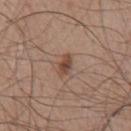<record>
<biopsy_status>not biopsied; imaged during a skin examination</biopsy_status>
<site>chest</site>
<image>
  <source>total-body photography crop</source>
  <field_of_view_mm>15</field_of_view_mm>
</image>
<lesion_size>
  <long_diameter_mm_approx>2.5</long_diameter_mm_approx>
</lesion_size>
<patient>
  <sex>male</sex>
  <age_approx>75</age_approx>
</patient>
<automated_metrics>
  <border_irregularity_0_10>2.0</border_irregularity_0_10>
  <color_variation_0_10>3.0</color_variation_0_10>
  <peripheral_color_asymmetry>0.5</peripheral_color_asymmetry>
  <nevus_likeness_0_100>85</nevus_likeness_0_100>
</automated_metrics>
</record>